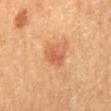• follow-up: no biopsy performed (imaged during a skin exam)
• subject: female, roughly 60 years of age
• tile lighting: cross-polarized
• imaging modality: total-body-photography crop, ~15 mm field of view
• size: about 3 mm
• site: the mid back
• automated lesion analysis: a shape eccentricity near 0.65 and a symmetry-axis asymmetry near 0.2